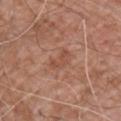A close-up tile cropped from a whole-body skin photograph, about 15 mm across.
The lesion is located on the left upper arm.
The subject is a male approximately 60 years of age.